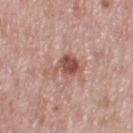Clinical impression: No biopsy was performed on this lesion — it was imaged during a full skin examination and was not determined to be concerning. Context: Cropped from a whole-body photographic skin survey; the tile spans about 15 mm. The lesion is located on the left thigh. The patient is a female aged approximately 50.